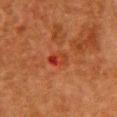Assessment: This lesion was catalogued during total-body skin photography and was not selected for biopsy. Clinical summary: A female patient, aged 48–52. Located on the chest. Cropped from a whole-body photographic skin survey; the tile spans about 15 mm.